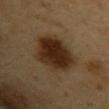Recorded during total-body skin imaging; not selected for excision or biopsy.
From the left upper arm.
A close-up tile cropped from a whole-body skin photograph, about 15 mm across.
A male patient, approximately 60 years of age.
Imaged with cross-polarized lighting.
An algorithmic analysis of the crop reported a lesion area of about 21 mm², an outline eccentricity of about 0.4 (0 = round, 1 = elongated), and two-axis asymmetry of about 0.15. The software also gave a lesion color around L≈27 a*≈16 b*≈27 in CIELAB, roughly 12 lightness units darker than nearby skin, and a lesion-to-skin contrast of about 12.5 (normalized; higher = more distinct). It also reported internal color variation of about 4.5 on a 0–10 scale.
Measured at roughly 6 mm in maximum diameter.